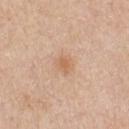Q: Is there a histopathology result?
A: imaged on a skin check; not biopsied
Q: Where on the body is the lesion?
A: the chest
Q: Who is the patient?
A: male, in their 60s
Q: Illumination type?
A: white-light
Q: What kind of image is this?
A: 15 mm crop, total-body photography